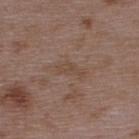notes=no biopsy performed (imaged during a skin exam); diameter=~4.5 mm (longest diameter); body site=the upper back; lighting=white-light; patient=male, approximately 50 years of age; acquisition=15 mm crop, total-body photography.